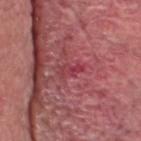biopsy status = imaged on a skin check; not biopsied | anatomic site = the chest | subject = male, in their mid-70s | image source = ~15 mm crop, total-body skin-cancer survey.